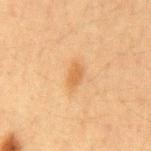The recorded lesion diameter is about 2.5 mm.
A male subject, in their mid-60s.
Captured under cross-polarized illumination.
The total-body-photography lesion software estimated a footprint of about 3.5 mm², an eccentricity of roughly 0.8, and two-axis asymmetry of about 0.35. The software also gave a mean CIELAB color near L≈53 a*≈20 b*≈37, a lesion–skin lightness drop of about 8, and a normalized lesion–skin contrast near 6. The analysis additionally found border irregularity of about 3 on a 0–10 scale, a color-variation rating of about 1/10, and a peripheral color-asymmetry measure near 0.5. And it measured a classifier nevus-likeness of about 55/100 and a detector confidence of about 100 out of 100 that the crop contains a lesion.
A roughly 15 mm field-of-view crop from a total-body skin photograph.
The lesion is located on the mid back.